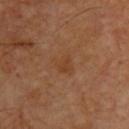No biopsy was performed on this lesion — it was imaged during a full skin examination and was not determined to be concerning. The recorded lesion diameter is about 3 mm. A male patient, aged around 60. A lesion tile, about 15 mm wide, cut from a 3D total-body photograph. From the back.